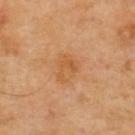Case summary:
- workup · imaged on a skin check; not biopsied